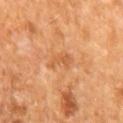Impression:
The lesion was photographed on a routine skin check and not biopsied; there is no pathology result.